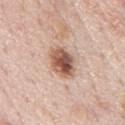Captured during whole-body skin photography for melanoma surveillance; the lesion was not biopsied. The lesion is on the back. The lesion's longest dimension is about 4.5 mm. The lesion-visualizer software estimated a lesion color around L≈57 a*≈21 b*≈28 in CIELAB, about 17 CIELAB-L* units darker than the surrounding skin, and a lesion-to-skin contrast of about 10.5 (normalized; higher = more distinct). And it measured a border-irregularity index near 2/10, a color-variation rating of about 9/10, and radial color variation of about 2.5. And it measured an automated nevus-likeness rating near 95 out of 100 and lesion-presence confidence of about 100/100. This is a white-light tile. Cropped from a whole-body photographic skin survey; the tile spans about 15 mm. A male patient about 75 years old.